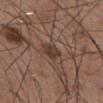Q: Was a biopsy performed?
A: catalogued during a skin exam; not biopsied
Q: How large is the lesion?
A: ~2.5 mm (longest diameter)
Q: What did automated image analysis measure?
A: a border-irregularity rating of about 2.5/10, a color-variation rating of about 2/10, and peripheral color asymmetry of about 1
Q: What kind of image is this?
A: 15 mm crop, total-body photography
Q: Lesion location?
A: the abdomen
Q: What are the patient's age and sex?
A: male, aged around 55